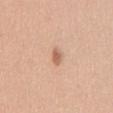follow-up = no biopsy performed (imaged during a skin exam) | image-analysis metrics = an average lesion color of about L≈62 a*≈22 b*≈32 (CIELAB) and about 11 CIELAB-L* units darker than the surrounding skin; a border-irregularity index near 3.5/10 and internal color variation of about 0 on a 0–10 scale | lighting = white-light illumination | image source = ~15 mm tile from a whole-body skin photo | anatomic site = the chest | patient = male, about 35 years old.